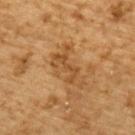Impression: This lesion was catalogued during total-body skin photography and was not selected for biopsy. Context: This is a cross-polarized tile. A male patient aged around 85 or older. Automated tile analysis of the lesion measured a lesion color around L≈43 a*≈18 b*≈35 in CIELAB and a normalized lesion–skin contrast near 6. The analysis additionally found border irregularity of about 8 on a 0–10 scale, a within-lesion color-variation index near 3/10, and a peripheral color-asymmetry measure near 1. Approximately 5.5 mm at its widest. A 15 mm crop from a total-body photograph taken for skin-cancer surveillance. On the back.